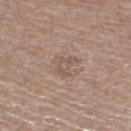Part of a total-body skin-imaging series; this lesion was reviewed on a skin check and was not flagged for biopsy. Automated image analysis of the tile measured a lesion color around L≈54 a*≈15 b*≈24 in CIELAB and a normalized lesion–skin contrast near 5. The software also gave border irregularity of about 5 on a 0–10 scale, a within-lesion color-variation index near 2.5/10, and peripheral color asymmetry of about 0.5. The analysis additionally found a detector confidence of about 100 out of 100 that the crop contains a lesion. A roughly 15 mm field-of-view crop from a total-body skin photograph. On the leg. Approximately 3 mm at its widest. The patient is a female aged around 55. Captured under white-light illumination.